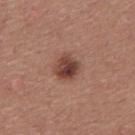Clinical impression:
Imaged during a routine full-body skin examination; the lesion was not biopsied and no histopathology is available.
Image and clinical context:
The tile uses white-light illumination. A male patient, aged around 40. Cropped from a whole-body photographic skin survey; the tile spans about 15 mm. From the upper back. About 3 mm across.